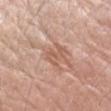{"biopsy_status": "not biopsied; imaged during a skin examination", "lesion_size": {"long_diameter_mm_approx": 3.0}, "site": "left forearm", "automated_metrics": {"area_mm2_approx": 5.0, "eccentricity": 0.7, "shape_asymmetry": 0.35, "cielab_L": 58, "cielab_a": 21, "cielab_b": 29, "vs_skin_darker_L": 8.0, "nevus_likeness_0_100": 0}, "lighting": "white-light", "image": {"source": "total-body photography crop", "field_of_view_mm": 15}, "patient": {"sex": "female", "age_approx": 65}}